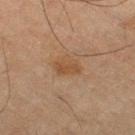Assessment:
This lesion was catalogued during total-body skin photography and was not selected for biopsy.
Acquisition and patient details:
A male patient, about 65 years old. On the right thigh. A close-up tile cropped from a whole-body skin photograph, about 15 mm across.